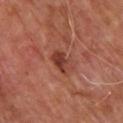Assessment: No biopsy was performed on this lesion — it was imaged during a full skin examination and was not determined to be concerning.